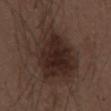biopsy_status: not biopsied; imaged during a skin examination
image:
  source: total-body photography crop
  field_of_view_mm: 15
lighting: white-light
patient:
  sex: male
  age_approx: 50
site: abdomen